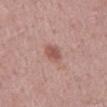The lesion was photographed on a routine skin check and not biopsied; there is no pathology result. A male subject, aged 58 to 62. A close-up tile cropped from a whole-body skin photograph, about 15 mm across. The lesion's longest dimension is about 3 mm. This is a white-light tile. The lesion is located on the mid back.